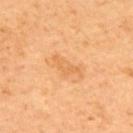No biopsy was performed on this lesion — it was imaged during a full skin examination and was not determined to be concerning. A male patient roughly 65 years of age. On the upper back. Longest diameter approximately 3.5 mm. A close-up tile cropped from a whole-body skin photograph, about 15 mm across. Automated tile analysis of the lesion measured border irregularity of about 6.5 on a 0–10 scale and a color-variation rating of about 1/10. The software also gave a nevus-likeness score of about 0/100 and lesion-presence confidence of about 100/100.